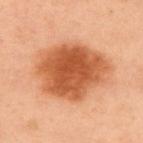Assessment: Imaged during a routine full-body skin examination; the lesion was not biopsied and no histopathology is available. Image and clinical context: The lesion is on the chest. Imaged with cross-polarized lighting. The lesion's longest dimension is about 8 mm. A close-up tile cropped from a whole-body skin photograph, about 15 mm across. Automated tile analysis of the lesion measured a footprint of about 37 mm², a shape eccentricity near 0.6, and a shape-asymmetry score of about 0.15 (0 = symmetric). A female patient about 55 years old.